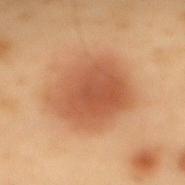Impression: The lesion was photographed on a routine skin check and not biopsied; there is no pathology result. Background: The patient is a male aged approximately 55. An algorithmic analysis of the crop reported an area of roughly 34 mm², an eccentricity of roughly 0.65, and a symmetry-axis asymmetry near 0.1. It also reported a lesion color around L≈45 a*≈21 b*≈31 in CIELAB, a lesion–skin lightness drop of about 9, and a normalized border contrast of about 7.5. The software also gave a border-irregularity rating of about 1.5/10, a color-variation rating of about 3.5/10, and peripheral color asymmetry of about 1. The software also gave an automated nevus-likeness rating near 100 out of 100 and a detector confidence of about 100 out of 100 that the crop contains a lesion. A region of skin cropped from a whole-body photographic capture, roughly 15 mm wide. The tile uses cross-polarized illumination. On the back. The recorded lesion diameter is about 7.5 mm.